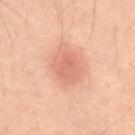notes: total-body-photography surveillance lesion; no biopsy | image: ~15 mm tile from a whole-body skin photo | automated metrics: a footprint of about 10 mm², an eccentricity of roughly 0.5, and a shape-asymmetry score of about 0.15 (0 = symmetric) | illumination: cross-polarized | patient: male, roughly 40 years of age | diameter: about 4 mm | site: the abdomen.